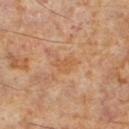Part of a total-body skin-imaging series; this lesion was reviewed on a skin check and was not flagged for biopsy.
The subject is a male aged around 60.
From the left lower leg.
Longest diameter approximately 3 mm.
A region of skin cropped from a whole-body photographic capture, roughly 15 mm wide.
Automated tile analysis of the lesion measured a lesion color around L≈53 a*≈20 b*≈35 in CIELAB and a lesion–skin lightness drop of about 5. It also reported an automated nevus-likeness rating near 0 out of 100 and a lesion-detection confidence of about 100/100.